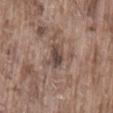Findings:
– workup — no biopsy performed (imaged during a skin exam)
– acquisition — ~15 mm tile from a whole-body skin photo
– site — the lower back
– tile lighting — white-light illumination
– patient — male, in their mid- to late 70s
– automated lesion analysis — a lesion area of about 4.5 mm², an outline eccentricity of about 0.7 (0 = round, 1 = elongated), and two-axis asymmetry of about 0.3; border irregularity of about 3 on a 0–10 scale and peripheral color asymmetry of about 1; an automated nevus-likeness rating near 0 out of 100 and a detector confidence of about 95 out of 100 that the crop contains a lesion
– diameter — ≈2.5 mm